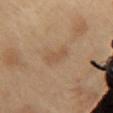workup: total-body-photography surveillance lesion; no biopsy
lighting: cross-polarized illumination
subject: female, approximately 55 years of age
imaging modality: total-body-photography crop, ~15 mm field of view
automated metrics: an area of roughly 4 mm², a shape eccentricity near 0.8, and a symmetry-axis asymmetry near 0.35; an average lesion color of about L≈53 a*≈17 b*≈33 (CIELAB), about 6 CIELAB-L* units darker than the surrounding skin, and a normalized lesion–skin contrast near 4.5; a nevus-likeness score of about 0/100 and lesion-presence confidence of about 100/100
site: the front of the torso